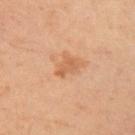  biopsy_status: not biopsied; imaged during a skin examination